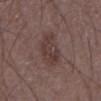Q: Was a biopsy performed?
A: total-body-photography surveillance lesion; no biopsy
Q: What did automated image analysis measure?
A: a lesion color around L≈37 a*≈17 b*≈18 in CIELAB, a lesion–skin lightness drop of about 7, and a normalized lesion–skin contrast near 6.5; an automated nevus-likeness rating near 0 out of 100
Q: Where on the body is the lesion?
A: the front of the torso
Q: What are the patient's age and sex?
A: male, aged 58 to 62
Q: What kind of image is this?
A: ~15 mm tile from a whole-body skin photo
Q: How was the tile lit?
A: white-light